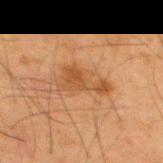Clinical impression:
Part of a total-body skin-imaging series; this lesion was reviewed on a skin check and was not flagged for biopsy.
Image and clinical context:
On the mid back. Measured at roughly 5 mm in maximum diameter. A 15 mm crop from a total-body photograph taken for skin-cancer surveillance. Automated tile analysis of the lesion measured an area of roughly 8 mm², a shape eccentricity near 0.9, and a symmetry-axis asymmetry near 0.65. It also reported a border-irregularity rating of about 7.5/10, a color-variation rating of about 2.5/10, and radial color variation of about 1. The software also gave lesion-presence confidence of about 100/100. A male patient, in their mid-30s.